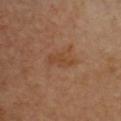<record>
<biopsy_status>not biopsied; imaged during a skin examination</biopsy_status>
<site>chest</site>
<lesion_size>
  <long_diameter_mm_approx>3.5</long_diameter_mm_approx>
</lesion_size>
<lighting>cross-polarized</lighting>
<image>
  <source>total-body photography crop</source>
  <field_of_view_mm>15</field_of_view_mm>
</image>
<patient>
  <sex>female</sex>
  <age_approx>55</age_approx>
</patient>
</record>